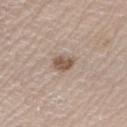Q: Illumination type?
A: white-light illumination
Q: What are the patient's age and sex?
A: male, aged 73–77
Q: How was this image acquired?
A: ~15 mm crop, total-body skin-cancer survey
Q: How large is the lesion?
A: about 2.5 mm
Q: Lesion location?
A: the left upper arm